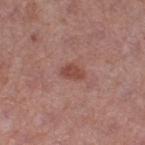Q: Was this lesion biopsied?
A: total-body-photography surveillance lesion; no biopsy
Q: How was this image acquired?
A: ~15 mm tile from a whole-body skin photo
Q: What did automated image analysis measure?
A: peripheral color asymmetry of about 0.5
Q: Where on the body is the lesion?
A: the right thigh
Q: Patient demographics?
A: female, aged 38 to 42